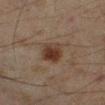Findings:
- workup — imaged on a skin check; not biopsied
- image — total-body-photography crop, ~15 mm field of view
- lesion size — about 3.5 mm
- site — the left lower leg
- subject — male, about 45 years old
- tile lighting — cross-polarized illumination